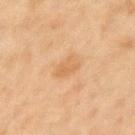The lesion was photographed on a routine skin check and not biopsied; there is no pathology result. The patient is a female about 40 years old. Automated tile analysis of the lesion measured a mean CIELAB color near L≈56 a*≈18 b*≈36, roughly 6 lightness units darker than nearby skin, and a lesion-to-skin contrast of about 5 (normalized; higher = more distinct). The software also gave an automated nevus-likeness rating near 0 out of 100. This image is a 15 mm lesion crop taken from a total-body photograph. Captured under cross-polarized illumination. From the left upper arm.